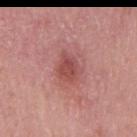– workup · catalogued during a skin exam; not biopsied
– image · ~15 mm crop, total-body skin-cancer survey
– anatomic site · the back
– lesion diameter · ≈4 mm
– lighting · white-light illumination
– subject · male, approximately 60 years of age
– image-analysis metrics · a mean CIELAB color near L≈49 a*≈29 b*≈24, about 10 CIELAB-L* units darker than the surrounding skin, and a lesion-to-skin contrast of about 7.5 (normalized; higher = more distinct); a classifier nevus-likeness of about 55/100 and a lesion-detection confidence of about 100/100